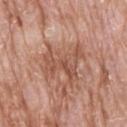Assessment:
The lesion was photographed on a routine skin check and not biopsied; there is no pathology result.
Image and clinical context:
This is a white-light tile. The recorded lesion diameter is about 5 mm. The patient is a male in their mid-70s. From the head or neck. A 15 mm close-up tile from a total-body photography series done for melanoma screening.